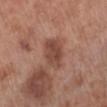Q: Is there a histopathology result?
A: imaged on a skin check; not biopsied
Q: Who is the patient?
A: male, aged approximately 70
Q: What lighting was used for the tile?
A: white-light
Q: Automated lesion metrics?
A: an area of roughly 9 mm²; a border-irregularity rating of about 1.5/10, a within-lesion color-variation index near 3/10, and radial color variation of about 1; a detector confidence of about 100 out of 100 that the crop contains a lesion
Q: What is the imaging modality?
A: ~15 mm tile from a whole-body skin photo
Q: Lesion size?
A: about 4 mm
Q: Lesion location?
A: the left lower leg